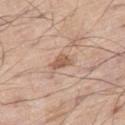  biopsy_status: not biopsied; imaged during a skin examination
  patient:
    sex: male
    age_approx: 70
  image:
    source: total-body photography crop
    field_of_view_mm: 15
  lesion_size:
    long_diameter_mm_approx: 2.5
  site: right thigh